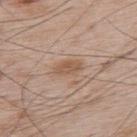Assessment: Captured during whole-body skin photography for melanoma surveillance; the lesion was not biopsied. Clinical summary: Cropped from a total-body skin-imaging series; the visible field is about 15 mm. This is a white-light tile. The recorded lesion diameter is about 3.5 mm. The patient is a male approximately 65 years of age. Located on the back.